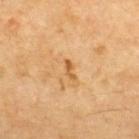Part of a total-body skin-imaging series; this lesion was reviewed on a skin check and was not flagged for biopsy. A male patient approximately 70 years of age. Captured under cross-polarized illumination. The lesion is on the back. A region of skin cropped from a whole-body photographic capture, roughly 15 mm wide.